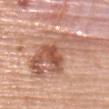A close-up tile cropped from a whole-body skin photograph, about 15 mm across.
The patient is a female about 60 years old.
Imaged with white-light lighting.
From the upper back.
The total-body-photography lesion software estimated a detector confidence of about 95 out of 100 that the crop contains a lesion.
The recorded lesion diameter is about 12 mm.